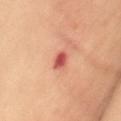Notes:
- biopsy status: no biopsy performed (imaged during a skin exam)
- subject: female, roughly 60 years of age
- body site: the abdomen
- lighting: cross-polarized
- lesion diameter: about 2.5 mm
- acquisition: total-body-photography crop, ~15 mm field of view
- automated lesion analysis: a lesion area of about 3.5 mm²